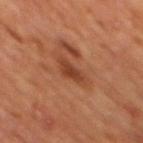follow-up: total-body-photography surveillance lesion; no biopsy
tile lighting: cross-polarized
imaging modality: 15 mm crop, total-body photography
patient: male, aged 63 to 67
body site: the mid back
automated lesion analysis: an area of roughly 5.5 mm², an eccentricity of roughly 0.85, and a shape-asymmetry score of about 0.35 (0 = symmetric); a border-irregularity rating of about 3.5/10 and peripheral color asymmetry of about 0.5